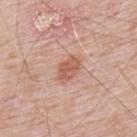This lesion was catalogued during total-body skin photography and was not selected for biopsy.